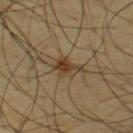Q: Is there a histopathology result?
A: no biopsy performed (imaged during a skin exam)
Q: What is the anatomic site?
A: the upper back
Q: Who is the patient?
A: male, about 45 years old
Q: How large is the lesion?
A: about 3 mm
Q: How was the tile lit?
A: cross-polarized illumination
Q: How was this image acquired?
A: total-body-photography crop, ~15 mm field of view